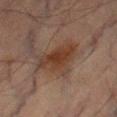From the leg. A male subject, aged 63–67. A lesion tile, about 15 mm wide, cut from a 3D total-body photograph.